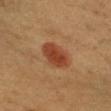| feature | finding |
|---|---|
| follow-up | total-body-photography surveillance lesion; no biopsy |
| acquisition | total-body-photography crop, ~15 mm field of view |
| subject | female, aged around 40 |
| location | the arm |
| automated metrics | an area of roughly 9 mm² and a shape eccentricity near 0.75; a lesion color around L≈36 a*≈23 b*≈30 in CIELAB and a normalized border contrast of about 8.5; border irregularity of about 2 on a 0–10 scale, a within-lesion color-variation index near 3/10, and peripheral color asymmetry of about 1; an automated nevus-likeness rating near 100 out of 100 and a lesion-detection confidence of about 100/100 |